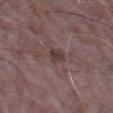The lesion is on the left forearm.
The lesion's longest dimension is about 2.5 mm.
A region of skin cropped from a whole-body photographic capture, roughly 15 mm wide.
A male subject in their mid-60s.
An algorithmic analysis of the crop reported an automated nevus-likeness rating near 0 out of 100 and a detector confidence of about 100 out of 100 that the crop contains a lesion.
The tile uses white-light illumination.